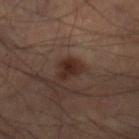follow-up = imaged on a skin check; not biopsied | image source = ~15 mm tile from a whole-body skin photo | lesion size = ≈2.5 mm | subject = male, aged 48–52 | TBP lesion metrics = a lesion color around L≈26 a*≈17 b*≈22 in CIELAB, about 9 CIELAB-L* units darker than the surrounding skin, and a normalized lesion–skin contrast near 9.5; a border-irregularity index near 3.5/10 and a peripheral color-asymmetry measure near 1 | anatomic site = the left leg | tile lighting = cross-polarized.